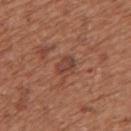Q: Was this lesion biopsied?
A: catalogued during a skin exam; not biopsied
Q: How large is the lesion?
A: ≈2.5 mm
Q: What is the anatomic site?
A: the back
Q: What are the patient's age and sex?
A: female, about 75 years old
Q: What is the imaging modality?
A: total-body-photography crop, ~15 mm field of view